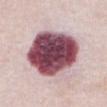follow-up=total-body-photography surveillance lesion; no biopsy | automated lesion analysis=an area of roughly 40 mm² and a symmetry-axis asymmetry near 0.1; a mean CIELAB color near L≈48 a*≈26 b*≈13, about 29 CIELAB-L* units darker than the surrounding skin, and a normalized lesion–skin contrast near 17.5; a border-irregularity index near 1.5/10, a within-lesion color-variation index near 9/10, and radial color variation of about 2.5; a nevus-likeness score of about 60/100 and a detector confidence of about 80 out of 100 that the crop contains a lesion | subject=female, approximately 65 years of age | anatomic site=the abdomen | lesion diameter=≈8.5 mm | acquisition=~15 mm crop, total-body skin-cancer survey | tile lighting=white-light illumination.